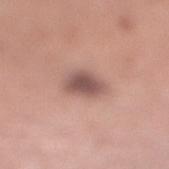The lesion is located on the right lower leg.
This image is a 15 mm lesion crop taken from a total-body photograph.
A female subject roughly 30 years of age.